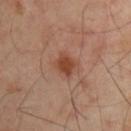The lesion was photographed on a routine skin check and not biopsied; there is no pathology result.
The patient is a male approximately 50 years of age.
A 15 mm crop from a total-body photograph taken for skin-cancer surveillance.
The lesion is located on the left arm.